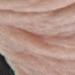Impression: Recorded during total-body skin imaging; not selected for excision or biopsy. Clinical summary: A lesion tile, about 15 mm wide, cut from a 3D total-body photograph. The lesion is on the left forearm. A female patient roughly 65 years of age.